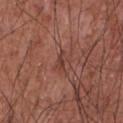workup = total-body-photography surveillance lesion; no biopsy
lesion diameter = ~2.5 mm (longest diameter)
anatomic site = the chest
image-analysis metrics = a footprint of about 3 mm², an eccentricity of roughly 0.85, and a symmetry-axis asymmetry near 0.4; a border-irregularity rating of about 4/10 and peripheral color asymmetry of about 0
subject = male, aged 73 to 77
illumination = white-light
image = ~15 mm crop, total-body skin-cancer survey